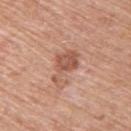lesion diameter = ≈5 mm
acquisition = ~15 mm tile from a whole-body skin photo
illumination = white-light illumination
patient = male, aged 58 to 62
site = the upper back